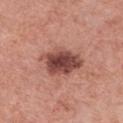biopsy_status: not biopsied; imaged during a skin examination
image:
  source: total-body photography crop
  field_of_view_mm: 15
lighting: white-light
lesion_size:
  long_diameter_mm_approx: 5.5
site: chest
patient:
  sex: female
  age_approx: 75